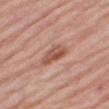Assessment:
Imaged during a routine full-body skin examination; the lesion was not biopsied and no histopathology is available.
Image and clinical context:
Located on the right thigh. This is a white-light tile. A close-up tile cropped from a whole-body skin photograph, about 15 mm across. Automated tile analysis of the lesion measured a lesion–skin lightness drop of about 11. It also reported border irregularity of about 2.5 on a 0–10 scale, a within-lesion color-variation index near 5/10, and a peripheral color-asymmetry measure near 1.5. The analysis additionally found a classifier nevus-likeness of about 60/100 and lesion-presence confidence of about 100/100. Measured at roughly 4 mm in maximum diameter. A male subject, aged around 80.